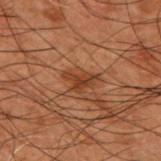notes: no biopsy performed (imaged during a skin exam) | size: ≈3.5 mm | patient: male, about 50 years old | image source: total-body-photography crop, ~15 mm field of view | site: the back.